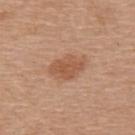<case>
  <biopsy_status>not biopsied; imaged during a skin examination</biopsy_status>
  <site>back</site>
  <lesion_size>
    <long_diameter_mm_approx>4.5</long_diameter_mm_approx>
  </lesion_size>
  <image>
    <source>total-body photography crop</source>
    <field_of_view_mm>15</field_of_view_mm>
  </image>
  <patient>
    <sex>male</sex>
    <age_approx>65</age_approx>
  </patient>
</case>